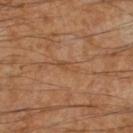Impression:
Captured during whole-body skin photography for melanoma surveillance; the lesion was not biopsied.
Clinical summary:
A 15 mm close-up extracted from a 3D total-body photography capture. The patient is a male in their 60s. The tile uses cross-polarized illumination. About 3 mm across. The lesion is located on the right lower leg. The total-body-photography lesion software estimated a normalized lesion–skin contrast near 4.5. The software also gave border irregularity of about 3 on a 0–10 scale, a within-lesion color-variation index near 0/10, and a peripheral color-asymmetry measure near 0. It also reported a classifier nevus-likeness of about 0/100 and lesion-presence confidence of about 70/100.